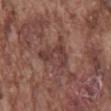Notes:
- notes: total-body-photography surveillance lesion; no biopsy
- diameter: ≈4.5 mm
- image source: ~15 mm crop, total-body skin-cancer survey
- patient: male, in their mid- to late 70s
- automated lesion analysis: a lesion color around L≈40 a*≈20 b*≈22 in CIELAB, roughly 8 lightness units darker than nearby skin, and a normalized lesion–skin contrast near 6.5; an automated nevus-likeness rating near 0 out of 100 and a detector confidence of about 55 out of 100 that the crop contains a lesion
- anatomic site: the back